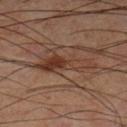No biopsy was performed on this lesion — it was imaged during a full skin examination and was not determined to be concerning. The lesion-visualizer software estimated a lesion area of about 11 mm² and two-axis asymmetry of about 0.5. It also reported a mean CIELAB color near L≈36 a*≈19 b*≈26 and a lesion–skin lightness drop of about 8. And it measured a classifier nevus-likeness of about 70/100 and a lesion-detection confidence of about 100/100. A 15 mm close-up extracted from a 3D total-body photography capture. Located on the leg. Longest diameter approximately 6.5 mm. The patient is a male aged 58–62.